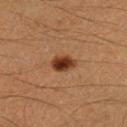Q: Was a biopsy performed?
A: no biopsy performed (imaged during a skin exam)
Q: Patient demographics?
A: male, roughly 40 years of age
Q: What kind of image is this?
A: total-body-photography crop, ~15 mm field of view
Q: What did automated image analysis measure?
A: a border-irregularity index near 2/10 and a within-lesion color-variation index near 4/10; a detector confidence of about 100 out of 100 that the crop contains a lesion
Q: Lesion location?
A: the right lower leg